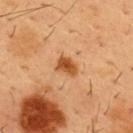  biopsy_status: not biopsied; imaged during a skin examination
  site: chest
  patient:
    sex: male
    age_approx: 55
  automated_metrics:
    area_mm2_approx: 4.0
    eccentricity: 0.7
    shape_asymmetry: 0.25
    cielab_L: 44
    cielab_a: 23
    cielab_b: 38
    vs_skin_darker_L: 12.0
    vs_skin_contrast_norm: 10.0
  image:
    source: total-body photography crop
    field_of_view_mm: 15
  lighting: cross-polarized
  lesion_size:
    long_diameter_mm_approx: 2.5